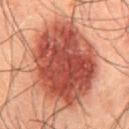Impression:
Imaged during a routine full-body skin examination; the lesion was not biopsied and no histopathology is available.
Acquisition and patient details:
A roughly 15 mm field-of-view crop from a total-body skin photograph. Measured at roughly 9 mm in maximum diameter. The lesion is on the abdomen. A male patient aged approximately 50.